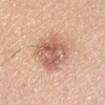Impression:
Captured during whole-body skin photography for melanoma surveillance; the lesion was not biopsied.
Acquisition and patient details:
The tile uses white-light illumination. This image is a 15 mm lesion crop taken from a total-body photograph. The total-body-photography lesion software estimated an area of roughly 19 mm² and two-axis asymmetry of about 0.2. The analysis additionally found a border-irregularity rating of about 2/10, a color-variation rating of about 6/10, and radial color variation of about 2. A male subject aged approximately 30. The recorded lesion diameter is about 6 mm. The lesion is located on the arm.